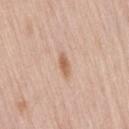- biopsy status: total-body-photography surveillance lesion; no biopsy
- subject: female, aged around 65
- lesion diameter: about 3 mm
- site: the right thigh
- lighting: white-light illumination
- image: ~15 mm crop, total-body skin-cancer survey